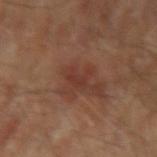Case summary:
* lesion size · ≈3 mm
* image source · ~15 mm tile from a whole-body skin photo
* automated metrics · a nevus-likeness score of about 5/100
* site · the left upper arm
* subject · male, about 65 years old
* tile lighting · cross-polarized illumination